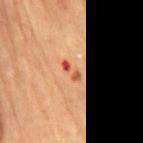biopsy status: imaged on a skin check; not biopsied